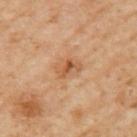No biopsy was performed on this lesion — it was imaged during a full skin examination and was not determined to be concerning. A 15 mm close-up tile from a total-body photography series done for melanoma screening. Longest diameter approximately 3 mm. Imaged with cross-polarized lighting. The lesion is located on the arm. The subject is a female aged approximately 60.